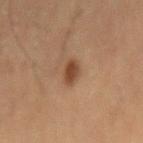Captured during whole-body skin photography for melanoma surveillance; the lesion was not biopsied. Imaged with cross-polarized lighting. An algorithmic analysis of the crop reported a footprint of about 4 mm², a shape eccentricity near 0.75, and two-axis asymmetry of about 0.25. And it measured a lesion–skin lightness drop of about 10 and a normalized lesion–skin contrast near 8.5. The analysis additionally found lesion-presence confidence of about 100/100. A 15 mm close-up tile from a total-body photography series done for melanoma screening. A male subject, approximately 60 years of age. The recorded lesion diameter is about 3 mm.